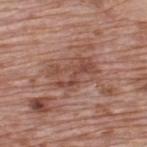No biopsy was performed on this lesion — it was imaged during a full skin examination and was not determined to be concerning.
Located on the upper back.
Measured at roughly 5 mm in maximum diameter.
This image is a 15 mm lesion crop taken from a total-body photograph.
Automated image analysis of the tile measured a shape eccentricity near 0.8 and two-axis asymmetry of about 0.3. And it measured a mean CIELAB color near L≈48 a*≈22 b*≈27 and about 8 CIELAB-L* units darker than the surrounding skin. It also reported border irregularity of about 4.5 on a 0–10 scale, a within-lesion color-variation index near 5.5/10, and a peripheral color-asymmetry measure near 2.
Captured under white-light illumination.
A male subject approximately 70 years of age.